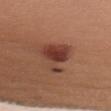{
  "biopsy_status": "not biopsied; imaged during a skin examination"
}